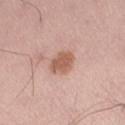Q: Was a biopsy performed?
A: no biopsy performed (imaged during a skin exam)
Q: Lesion size?
A: ≈3 mm
Q: Illumination type?
A: white-light
Q: Patient demographics?
A: male, approximately 70 years of age
Q: How was this image acquired?
A: ~15 mm tile from a whole-body skin photo
Q: Lesion location?
A: the right thigh